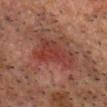Part of a total-body skin-imaging series; this lesion was reviewed on a skin check and was not flagged for biopsy.
Located on the head or neck.
An algorithmic analysis of the crop reported a lesion color around L≈29 a*≈22 b*≈21 in CIELAB, a lesion–skin lightness drop of about 6, and a lesion-to-skin contrast of about 6.5 (normalized; higher = more distinct).
A male patient aged 58 to 62.
This image is a 15 mm lesion crop taken from a total-body photograph.